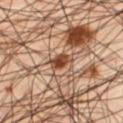Acquisition and patient details:
From the left thigh. An algorithmic analysis of the crop reported a lesion area of about 4.5 mm², a shape eccentricity near 0.85, and a shape-asymmetry score of about 0.3 (0 = symmetric). The software also gave border irregularity of about 3 on a 0–10 scale, a within-lesion color-variation index near 4.5/10, and peripheral color asymmetry of about 1.5. The software also gave a lesion-detection confidence of about 95/100. Imaged with cross-polarized lighting. A male subject, aged 43 to 47. About 3.5 mm across. A 15 mm close-up extracted from a 3D total-body photography capture.